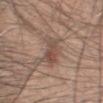biopsy status — total-body-photography surveillance lesion; no biopsy
body site — the head or neck
patient — male, aged 33 to 37
acquisition — 15 mm crop, total-body photography